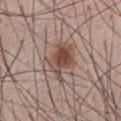A male subject, aged 53–57.
Automated tile analysis of the lesion measured an area of roughly 12 mm², an eccentricity of roughly 0.8, and a symmetry-axis asymmetry near 0.35. The analysis additionally found border irregularity of about 4.5 on a 0–10 scale and internal color variation of about 8.5 on a 0–10 scale.
The recorded lesion diameter is about 5 mm.
A 15 mm close-up extracted from a 3D total-body photography capture.
On the front of the torso.
Imaged with white-light lighting.